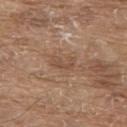The lesion-visualizer software estimated a lesion area of about 4 mm², an eccentricity of roughly 0.85, and a shape-asymmetry score of about 0.4 (0 = symmetric). It also reported a lesion color around L≈49 a*≈18 b*≈29 in CIELAB and a lesion-to-skin contrast of about 5 (normalized; higher = more distinct). The analysis additionally found a border-irregularity rating of about 4.5/10 and peripheral color asymmetry of about 0. The analysis additionally found a nevus-likeness score of about 0/100 and lesion-presence confidence of about 100/100. The subject is a male aged 78 to 82. About 3 mm across. The lesion is on the upper back. A lesion tile, about 15 mm wide, cut from a 3D total-body photograph. Imaged with white-light lighting.